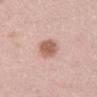| field | value |
|---|---|
| follow-up | total-body-photography surveillance lesion; no biopsy |
| subject | female, aged approximately 65 |
| anatomic site | the mid back |
| image source | ~15 mm tile from a whole-body skin photo |
| TBP lesion metrics | border irregularity of about 1.5 on a 0–10 scale and radial color variation of about 1 |
| illumination | white-light |